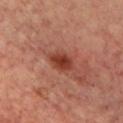Part of a total-body skin-imaging series; this lesion was reviewed on a skin check and was not flagged for biopsy. The patient is a female aged 43–47. The lesion is on the chest. The total-body-photography lesion software estimated a footprint of about 6 mm², a shape eccentricity near 0.75, and two-axis asymmetry of about 0.15. The software also gave a mean CIELAB color near L≈39 a*≈29 b*≈30, a lesion–skin lightness drop of about 12, and a normalized border contrast of about 9.5. The lesion's longest dimension is about 3.5 mm. Cropped from a total-body skin-imaging series; the visible field is about 15 mm.